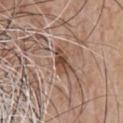Case summary:
- workup — imaged on a skin check; not biopsied
- lighting — white-light
- anatomic site — the front of the torso
- size — ≈3 mm
- automated lesion analysis — a lesion color around L≈46 a*≈20 b*≈29 in CIELAB, roughly 12 lightness units darker than nearby skin, and a normalized lesion–skin contrast near 9.5; a border-irregularity index near 2.5/10, a color-variation rating of about 3.5/10, and peripheral color asymmetry of about 1.5
- acquisition — ~15 mm tile from a whole-body skin photo
- subject — male, in their mid- to late 50s